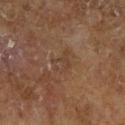A male subject, roughly 65 years of age.
Approximately 3 mm at its widest.
A lesion tile, about 15 mm wide, cut from a 3D total-body photograph.